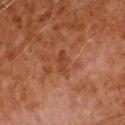follow-up: no biopsy performed (imaged during a skin exam)
patient: male, about 70 years old
anatomic site: the right upper arm
image source: ~15 mm crop, total-body skin-cancer survey